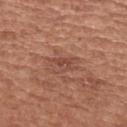imaging modality: ~15 mm crop, total-body skin-cancer survey | tile lighting: white-light illumination | patient: male, in their mid- to late 50s | TBP lesion metrics: a lesion area of about 3 mm², a shape eccentricity near 0.75, and a shape-asymmetry score of about 0.45 (0 = symmetric); a lesion color around L≈46 a*≈24 b*≈28 in CIELAB, roughly 7 lightness units darker than nearby skin, and a normalized lesion–skin contrast near 5.5; a border-irregularity index near 4.5/10, a color-variation rating of about 2.5/10, and a peripheral color-asymmetry measure near 0.5 | lesion diameter: ≈2.5 mm | body site: the upper back.